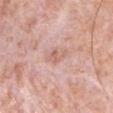Q: Was a biopsy performed?
A: total-body-photography surveillance lesion; no biopsy
Q: What are the patient's age and sex?
A: male, in their 80s
Q: Lesion location?
A: the arm
Q: How large is the lesion?
A: ~2.5 mm (longest diameter)
Q: What kind of image is this?
A: ~15 mm crop, total-body skin-cancer survey
Q: How was the tile lit?
A: white-light